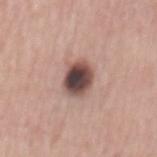Case summary:
- location · the mid back
- patient · male, in their mid-60s
- image · ~15 mm tile from a whole-body skin photo